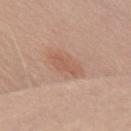Acquisition and patient details:
The lesion is located on the right upper arm. Measured at roughly 3 mm in maximum diameter. The patient is a female aged around 45. The tile uses white-light illumination. A close-up tile cropped from a whole-body skin photograph, about 15 mm across.